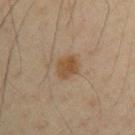{"biopsy_status": "not biopsied; imaged during a skin examination", "lesion_size": {"long_diameter_mm_approx": 3.0}, "site": "left upper arm", "patient": {"sex": "male", "age_approx": 35}, "image": {"source": "total-body photography crop", "field_of_view_mm": 15}}